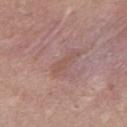Impression:
Part of a total-body skin-imaging series; this lesion was reviewed on a skin check and was not flagged for biopsy.
Clinical summary:
Measured at roughly 3 mm in maximum diameter. Automated image analysis of the tile measured an average lesion color of about L≈52 a*≈20 b*≈24 (CIELAB), about 5 CIELAB-L* units darker than the surrounding skin, and a lesion-to-skin contrast of about 5 (normalized; higher = more distinct). And it measured a border-irregularity rating of about 5.5/10 and a within-lesion color-variation index near 0/10. The analysis additionally found a classifier nevus-likeness of about 0/100 and a lesion-detection confidence of about 95/100. A lesion tile, about 15 mm wide, cut from a 3D total-body photograph. The tile uses white-light illumination. Located on the mid back. A male subject, about 25 years old.